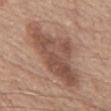Clinical impression: The lesion was tiled from a total-body skin photograph and was not biopsied. Clinical summary: A male patient, in their mid- to late 70s. An algorithmic analysis of the crop reported an area of roughly 32 mm², a shape eccentricity near 0.85, and a symmetry-axis asymmetry near 0.4. It also reported an automated nevus-likeness rating near 20 out of 100. The lesion is located on the chest. About 10 mm across. This image is a 15 mm lesion crop taken from a total-body photograph. This is a white-light tile.